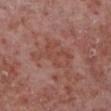Recorded during total-body skin imaging; not selected for excision or biopsy.
A close-up tile cropped from a whole-body skin photograph, about 15 mm across.
From the left lower leg.
A male patient aged around 55.